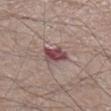Q: Is there a histopathology result?
A: catalogued during a skin exam; not biopsied
Q: What is the anatomic site?
A: the right lower leg
Q: Lesion size?
A: about 3 mm
Q: Who is the patient?
A: male, aged around 60
Q: What is the imaging modality?
A: total-body-photography crop, ~15 mm field of view
Q: What lighting was used for the tile?
A: white-light illumination